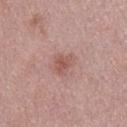{"biopsy_status": "not biopsied; imaged during a skin examination", "lighting": "white-light", "image": {"source": "total-body photography crop", "field_of_view_mm": 15}, "patient": {"sex": "male", "age_approx": 30}, "automated_metrics": {"cielab_L": 54, "cielab_a": 23, "cielab_b": 24, "vs_skin_darker_L": 9.0, "vs_skin_contrast_norm": 6.5, "border_irregularity_0_10": 4.0, "peripheral_color_asymmetry": 1.0}, "lesion_size": {"long_diameter_mm_approx": 2.5}}